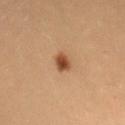The subject is a female aged approximately 20.
The lesion-visualizer software estimated a border-irregularity index near 2/10 and a peripheral color-asymmetry measure near 1. The software also gave an automated nevus-likeness rating near 100 out of 100 and a lesion-detection confidence of about 100/100.
The lesion is on the head or neck.
Cropped from a whole-body photographic skin survey; the tile spans about 15 mm.